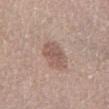Clinical impression:
Recorded during total-body skin imaging; not selected for excision or biopsy.